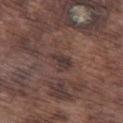| field | value |
|---|---|
| illumination | white-light |
| anatomic site | the left thigh |
| size | ≈3 mm |
| patient | male, aged around 75 |
| image source | 15 mm crop, total-body photography |
| image-analysis metrics | a lesion–skin lightness drop of about 7 and a normalized lesion–skin contrast near 7.5; a nevus-likeness score of about 5/100 and a detector confidence of about 100 out of 100 that the crop contains a lesion |